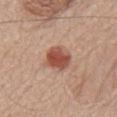Clinical impression:
The lesion was photographed on a routine skin check and not biopsied; there is no pathology result.
Acquisition and patient details:
This is a white-light tile. The lesion's longest dimension is about 4 mm. A close-up tile cropped from a whole-body skin photograph, about 15 mm across. A male subject about 80 years old. The lesion-visualizer software estimated a lesion area of about 9 mm² and an eccentricity of roughly 0.7. And it measured radial color variation of about 1. On the chest.